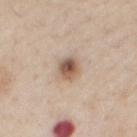The lesion was photographed on a routine skin check and not biopsied; there is no pathology result.